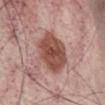| key | value |
|---|---|
| biopsy status | total-body-photography surveillance lesion; no biopsy |
| patient | male, approximately 55 years of age |
| lighting | white-light |
| location | the abdomen |
| size | about 6 mm |
| TBP lesion metrics | an area of roughly 19 mm², a shape eccentricity near 0.75, and two-axis asymmetry of about 0.15; a lesion color around L≈50 a*≈24 b*≈27 in CIELAB and roughly 14 lightness units darker than nearby skin; an automated nevus-likeness rating near 70 out of 100 |
| acquisition | ~15 mm crop, total-body skin-cancer survey |